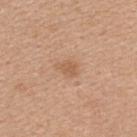The lesion was tiled from a total-body skin photograph and was not biopsied. The lesion is located on the upper back. A 15 mm close-up tile from a total-body photography series done for melanoma screening. Automated tile analysis of the lesion measured a lesion color around L≈58 a*≈20 b*≈34 in CIELAB and about 8 CIELAB-L* units darker than the surrounding skin. The software also gave border irregularity of about 3 on a 0–10 scale, a within-lesion color-variation index near 1.5/10, and a peripheral color-asymmetry measure near 0.5. About 2.5 mm across. Imaged with white-light lighting. The patient is a female in their 40s.